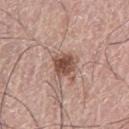Notes:
• biopsy status · total-body-photography surveillance lesion; no biopsy
• size · about 3 mm
• automated lesion analysis · a footprint of about 6.5 mm², an eccentricity of roughly 0.35, and two-axis asymmetry of about 0.2; an average lesion color of about L≈49 a*≈21 b*≈25 (CIELAB), about 14 CIELAB-L* units darker than the surrounding skin, and a normalized lesion–skin contrast near 10
• patient · male, aged around 75
• location · the leg
• image source · ~15 mm crop, total-body skin-cancer survey